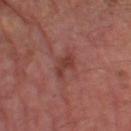{"biopsy_status": "not biopsied; imaged during a skin examination", "patient": {"sex": "male", "age_approx": 65}, "image": {"source": "total-body photography crop", "field_of_view_mm": 15}, "site": "left thigh", "lesion_size": {"long_diameter_mm_approx": 3.0}, "lighting": "cross-polarized"}